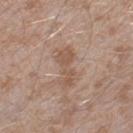Q: What kind of image is this?
A: ~15 mm crop, total-body skin-cancer survey
Q: What lighting was used for the tile?
A: white-light illumination
Q: Where on the body is the lesion?
A: the right lower leg
Q: Patient demographics?
A: male, aged around 45
Q: What did automated image analysis measure?
A: a footprint of about 7.5 mm², an outline eccentricity of about 0.9 (0 = round, 1 = elongated), and a shape-asymmetry score of about 0.4 (0 = symmetric); a classifier nevus-likeness of about 0/100 and a detector confidence of about 100 out of 100 that the crop contains a lesion
Q: What is the lesion's diameter?
A: ≈4.5 mm